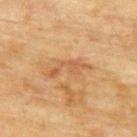Captured during whole-body skin photography for melanoma surveillance; the lesion was not biopsied.
A female subject, about 80 years old.
Located on the upper back.
A lesion tile, about 15 mm wide, cut from a 3D total-body photograph.